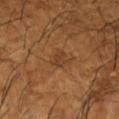A close-up tile cropped from a whole-body skin photograph, about 15 mm across.
A male subject, approximately 65 years of age.
Located on the left forearm.